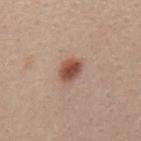Clinical impression:
The lesion was photographed on a routine skin check and not biopsied; there is no pathology result.
Acquisition and patient details:
Cropped from a whole-body photographic skin survey; the tile spans about 15 mm. A female patient, in their 40s. The lesion is located on the mid back.